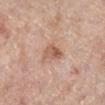Case summary:
• biopsy status — imaged on a skin check; not biopsied
• imaging modality — total-body-photography crop, ~15 mm field of view
• body site — the right lower leg
• subject — female, roughly 65 years of age